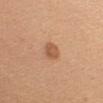biopsy_status: not biopsied; imaged during a skin examination
site: right upper arm
image:
  source: total-body photography crop
  field_of_view_mm: 15
patient:
  sex: female
  age_approx: 45